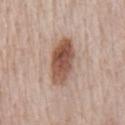Impression: The lesion was photographed on a routine skin check and not biopsied; there is no pathology result. Clinical summary: From the chest. A lesion tile, about 15 mm wide, cut from a 3D total-body photograph. The subject is a male aged around 80.